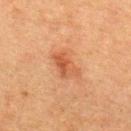follow-up — imaged on a skin check; not biopsied
diameter — ~4 mm (longest diameter)
illumination — cross-polarized
subject — female, in their mid- to late 50s
automated metrics — an eccentricity of roughly 0.8 and a shape-asymmetry score of about 0.3 (0 = symmetric); internal color variation of about 4 on a 0–10 scale
anatomic site — the upper back
acquisition — total-body-photography crop, ~15 mm field of view